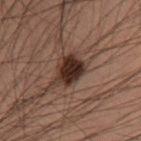<record>
<biopsy_status>not biopsied; imaged during a skin examination</biopsy_status>
<lesion_size>
  <long_diameter_mm_approx>3.0</long_diameter_mm_approx>
</lesion_size>
<patient>
  <sex>male</sex>
  <age_approx>55</age_approx>
</patient>
<site>right lower leg</site>
<image>
  <source>total-body photography crop</source>
  <field_of_view_mm>15</field_of_view_mm>
</image>
<lighting>cross-polarized</lighting>
</record>